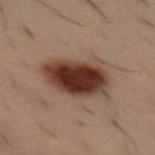Q: Is there a histopathology result?
A: catalogued during a skin exam; not biopsied
Q: How was the tile lit?
A: cross-polarized illumination
Q: Lesion size?
A: about 6 mm
Q: What kind of image is this?
A: ~15 mm crop, total-body skin-cancer survey
Q: Lesion location?
A: the leg
Q: Patient demographics?
A: male, aged 48 to 52
Q: Automated lesion metrics?
A: a lesion area of about 21 mm², a shape eccentricity near 0.8, and two-axis asymmetry of about 0.2; a mean CIELAB color near L≈27 a*≈16 b*≈20, about 14 CIELAB-L* units darker than the surrounding skin, and a lesion-to-skin contrast of about 13.5 (normalized; higher = more distinct)